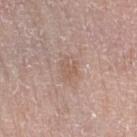biopsy status=imaged on a skin check; not biopsied
anatomic site=the arm
patient=female, in their mid-60s
diameter=~3 mm (longest diameter)
image source=15 mm crop, total-body photography
lighting=white-light illumination
image-analysis metrics=a footprint of about 5 mm² and two-axis asymmetry of about 0.3; a within-lesion color-variation index near 2.5/10 and a peripheral color-asymmetry measure near 1; an automated nevus-likeness rating near 0 out of 100 and lesion-presence confidence of about 100/100